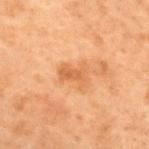Captured during whole-body skin photography for melanoma surveillance; the lesion was not biopsied. The lesion is on the upper back. Cropped from a total-body skin-imaging series; the visible field is about 15 mm. A male patient about 70 years old.